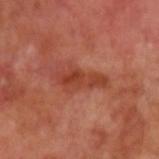Q: Was a biopsy performed?
A: catalogued during a skin exam; not biopsied
Q: Who is the patient?
A: male, aged 68 to 72
Q: How was the tile lit?
A: cross-polarized illumination
Q: Lesion location?
A: the left upper arm
Q: Automated lesion metrics?
A: a footprint of about 8.5 mm² and an outline eccentricity of about 0.95 (0 = round, 1 = elongated); an average lesion color of about L≈42 a*≈30 b*≈32 (CIELAB); an automated nevus-likeness rating near 0 out of 100
Q: What kind of image is this?
A: 15 mm crop, total-body photography